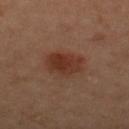Assessment:
This lesion was catalogued during total-body skin photography and was not selected for biopsy.
Image and clinical context:
The tile uses cross-polarized illumination. The lesion is located on the upper back. Cropped from a total-body skin-imaging series; the visible field is about 15 mm. Approximately 4.5 mm at its widest. The subject is a female roughly 45 years of age.